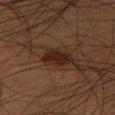Recorded during total-body skin imaging; not selected for excision or biopsy.
The lesion is on the right thigh.
Imaged with cross-polarized lighting.
The total-body-photography lesion software estimated border irregularity of about 2.5 on a 0–10 scale, a within-lesion color-variation index near 2.5/10, and peripheral color asymmetry of about 1. And it measured an automated nevus-likeness rating near 85 out of 100.
Cropped from a whole-body photographic skin survey; the tile spans about 15 mm.
Measured at roughly 4 mm in maximum diameter.
A male subject, about 50 years old.